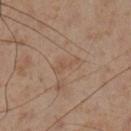Q: Was this lesion biopsied?
A: catalogued during a skin exam; not biopsied
Q: How was this image acquired?
A: total-body-photography crop, ~15 mm field of view
Q: Lesion location?
A: the left lower leg
Q: Who is the patient?
A: male, aged around 55